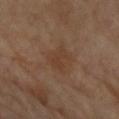* notes · imaged on a skin check; not biopsied
* anatomic site · the left forearm
* lesion diameter · ≈3 mm
* patient · female, about 70 years old
* illumination · cross-polarized
* acquisition · ~15 mm tile from a whole-body skin photo